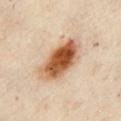{
  "biopsy_status": "not biopsied; imaged during a skin examination",
  "site": "chest",
  "patient": {
    "sex": "female",
    "age_approx": 60
  },
  "image": {
    "source": "total-body photography crop",
    "field_of_view_mm": 15
  }
}